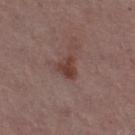• biopsy status — imaged on a skin check; not biopsied
• subject — female, roughly 55 years of age
• body site — the right thigh
• image — total-body-photography crop, ~15 mm field of view
• lesion diameter — about 2.5 mm
• lighting — white-light